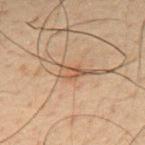Case summary:
• workup — total-body-photography surveillance lesion; no biopsy
• subject — male, about 50 years old
• automated lesion analysis — a border-irregularity index near 3/10, a within-lesion color-variation index near 4/10, and a peripheral color-asymmetry measure near 1.5; a lesion-detection confidence of about 95/100
• lesion size — ≈3 mm
• location — the mid back
• image source — ~15 mm crop, total-body skin-cancer survey